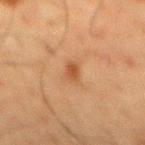Q: Is there a histopathology result?
A: catalogued during a skin exam; not biopsied
Q: How was this image acquired?
A: total-body-photography crop, ~15 mm field of view
Q: Where on the body is the lesion?
A: the back
Q: Illumination type?
A: cross-polarized
Q: What are the patient's age and sex?
A: male, aged approximately 60